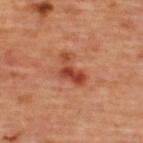workup=total-body-photography surveillance lesion; no biopsy
subject=female, in their mid-40s
image source=total-body-photography crop, ~15 mm field of view
location=the upper back
TBP lesion metrics=an area of roughly 6 mm², an eccentricity of roughly 0.85, and two-axis asymmetry of about 0.6; an automated nevus-likeness rating near 0 out of 100
lesion size=~4 mm (longest diameter)
illumination=cross-polarized illumination